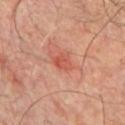Clinical impression:
Captured during whole-body skin photography for melanoma surveillance; the lesion was not biopsied.
Image and clinical context:
The lesion is on the chest. A 15 mm crop from a total-body photograph taken for skin-cancer surveillance. About 2.5 mm across. An algorithmic analysis of the crop reported a footprint of about 4 mm², an eccentricity of roughly 0.7, and a symmetry-axis asymmetry near 0.3. And it measured border irregularity of about 3 on a 0–10 scale and radial color variation of about 0.5. It also reported a classifier nevus-likeness of about 0/100 and a detector confidence of about 100 out of 100 that the crop contains a lesion. A male subject aged approximately 55.